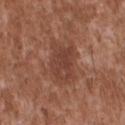Captured during whole-body skin photography for melanoma surveillance; the lesion was not biopsied. From the upper back. A region of skin cropped from a whole-body photographic capture, roughly 15 mm wide. The recorded lesion diameter is about 5 mm. Imaged with white-light lighting. A male subject, approximately 45 years of age.